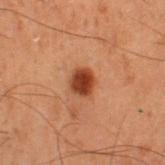This lesion was catalogued during total-body skin photography and was not selected for biopsy.